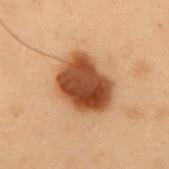{"biopsy_status": "not biopsied; imaged during a skin examination", "site": "mid back", "image": {"source": "total-body photography crop", "field_of_view_mm": 15}, "patient": {"sex": "male", "age_approx": 55}}